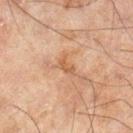biopsy status = no biopsy performed (imaged during a skin exam); site = the right lower leg; automated lesion analysis = an average lesion color of about L≈47 a*≈18 b*≈31 (CIELAB), about 7 CIELAB-L* units darker than the surrounding skin, and a normalized lesion–skin contrast near 6; acquisition = total-body-photography crop, ~15 mm field of view; patient = male, aged 43–47; lesion diameter = about 2.5 mm.